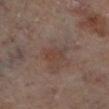{
  "biopsy_status": "not biopsied; imaged during a skin examination",
  "site": "left lower leg",
  "patient": {
    "sex": "female",
    "age_approx": 60
  },
  "lighting": "cross-polarized",
  "image": {
    "source": "total-body photography crop",
    "field_of_view_mm": 15
  },
  "automated_metrics": {
    "cielab_L": 40,
    "cielab_a": 17,
    "cielab_b": 24,
    "vs_skin_darker_L": 5.0,
    "vs_skin_contrast_norm": 5.0
  }
}